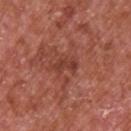  biopsy_status: not biopsied; imaged during a skin examination
  patient:
    sex: male
    age_approx: 65
  image:
    source: total-body photography crop
    field_of_view_mm: 15
  lighting: white-light
  site: upper back
  lesion_size:
    long_diameter_mm_approx: 3.0